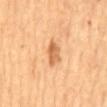Assessment: The lesion was photographed on a routine skin check and not biopsied; there is no pathology result. Background: A 15 mm close-up tile from a total-body photography series done for melanoma screening. An algorithmic analysis of the crop reported an area of roughly 5 mm², an eccentricity of roughly 0.85, and a symmetry-axis asymmetry near 0.3. It also reported an average lesion color of about L≈63 a*≈24 b*≈42 (CIELAB), a lesion–skin lightness drop of about 12, and a lesion-to-skin contrast of about 7.5 (normalized; higher = more distinct). The software also gave an automated nevus-likeness rating near 65 out of 100 and lesion-presence confidence of about 100/100. A male subject, aged 58 to 62. This is a cross-polarized tile. Approximately 3.5 mm at its widest. Located on the mid back.